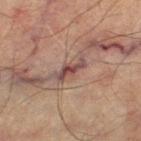Recorded during total-body skin imaging; not selected for excision or biopsy.
The lesion is on the left thigh.
This image is a 15 mm lesion crop taken from a total-body photograph.
Longest diameter approximately 3.5 mm.
A male subject aged approximately 75.
Imaged with cross-polarized lighting.